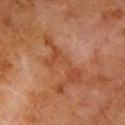Q: How was the tile lit?
A: cross-polarized illumination
Q: What is the anatomic site?
A: the chest
Q: How large is the lesion?
A: ≈7.5 mm
Q: What did automated image analysis measure?
A: a lesion color around L≈36 a*≈21 b*≈29 in CIELAB, roughly 6 lightness units darker than nearby skin, and a lesion-to-skin contrast of about 5.5 (normalized; higher = more distinct); a lesion-detection confidence of about 60/100
Q: Patient demographics?
A: male, roughly 80 years of age
Q: How was this image acquired?
A: 15 mm crop, total-body photography